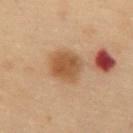Impression:
The lesion was photographed on a routine skin check and not biopsied; there is no pathology result.
Acquisition and patient details:
From the abdomen. The subject is a male about 55 years old. Imaged with cross-polarized lighting. A 15 mm close-up tile from a total-body photography series done for melanoma screening. The lesion's longest dimension is about 3.5 mm.